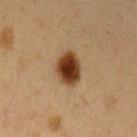Assessment: Imaged during a routine full-body skin examination; the lesion was not biopsied and no histopathology is available. Context: A close-up tile cropped from a whole-body skin photograph, about 15 mm across. The lesion is located on the left upper arm. Imaged with cross-polarized lighting. A female subject aged 38–42.